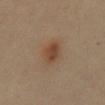{"biopsy_status": "not biopsied; imaged during a skin examination", "lesion_size": {"long_diameter_mm_approx": 4.0}, "patient": {"sex": "female", "age_approx": 35}, "automated_metrics": {"area_mm2_approx": 7.0, "eccentricity": 0.8, "shape_asymmetry": 0.2, "cielab_L": 43, "cielab_a": 18, "cielab_b": 29, "vs_skin_darker_L": 9.0, "vs_skin_contrast_norm": 7.5, "border_irregularity_0_10": 2.5, "color_variation_0_10": 3.0, "peripheral_color_asymmetry": 1.0}, "image": {"source": "total-body photography crop", "field_of_view_mm": 15}, "lighting": "cross-polarized", "site": "abdomen"}